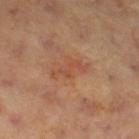notes: no biopsy performed (imaged during a skin exam) | imaging modality: 15 mm crop, total-body photography | site: the right thigh | patient: female, about 40 years old | size: ~4 mm (longest diameter) | illumination: cross-polarized illumination | image-analysis metrics: radial color variation of about 0.5.